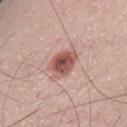Part of a total-body skin-imaging series; this lesion was reviewed on a skin check and was not flagged for biopsy. The patient is a male aged 58–62. The lesion-visualizer software estimated a footprint of about 8 mm² and an outline eccentricity of about 0.75 (0 = round, 1 = elongated). And it measured a lesion color around L≈53 a*≈24 b*≈24 in CIELAB, a lesion–skin lightness drop of about 15, and a lesion-to-skin contrast of about 10 (normalized; higher = more distinct). It also reported a color-variation rating of about 5.5/10 and radial color variation of about 2. The lesion is located on the abdomen. A 15 mm crop from a total-body photograph taken for skin-cancer surveillance. Longest diameter approximately 4 mm.